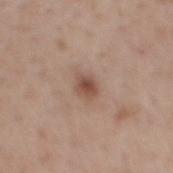Findings:
• biopsy status · no biopsy performed (imaged during a skin exam)
• TBP lesion metrics · an outline eccentricity of about 0.55 (0 = round, 1 = elongated); a classifier nevus-likeness of about 80/100 and a detector confidence of about 100 out of 100 that the crop contains a lesion
• diameter · ≈2.5 mm
• tile lighting · white-light illumination
• acquisition · total-body-photography crop, ~15 mm field of view
• anatomic site · the mid back
• patient · male, approximately 55 years of age